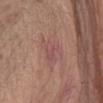notes = catalogued during a skin exam; not biopsied | image source = ~15 mm crop, total-body skin-cancer survey | automated lesion analysis = a footprint of about 7.5 mm² and a symmetry-axis asymmetry near 0.35 | body site = the left forearm | diameter = ≈4 mm | patient = male, aged around 70 | lighting = white-light illumination.